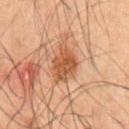Findings:
- location — the abdomen
- subject — male, in their 50s
- image source — total-body-photography crop, ~15 mm field of view
- lesion diameter — ≈4.5 mm